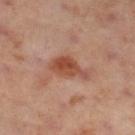Findings:
• diameter: ~4.5 mm (longest diameter)
• patient: female, aged 53–57
• lighting: cross-polarized illumination
• site: the right lower leg
• imaging modality: ~15 mm crop, total-body skin-cancer survey